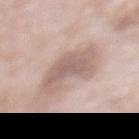Notes:
• patient · male, aged approximately 55
• site · the abdomen
• illumination · white-light illumination
• image source · 15 mm crop, total-body photography